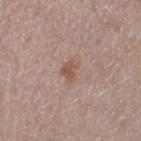Imaged during a routine full-body skin examination; the lesion was not biopsied and no histopathology is available. The lesion is located on the left thigh. An algorithmic analysis of the crop reported a lesion area of about 4 mm², an eccentricity of roughly 0.75, and two-axis asymmetry of about 0.4. Approximately 2.5 mm at its widest. The patient is a male about 65 years old. The tile uses white-light illumination. Cropped from a whole-body photographic skin survey; the tile spans about 15 mm.